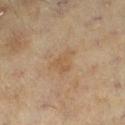• workup: catalogued during a skin exam; not biopsied
• lighting: cross-polarized
• subject: female, about 60 years old
• imaging modality: ~15 mm crop, total-body skin-cancer survey
• body site: the left lower leg
• TBP lesion metrics: an outline eccentricity of about 0.75 (0 = round, 1 = elongated) and a symmetry-axis asymmetry near 0.45; an average lesion color of about L≈50 a*≈15 b*≈31 (CIELAB) and a lesion-to-skin contrast of about 5 (normalized; higher = more distinct)
• lesion diameter: about 3.5 mm